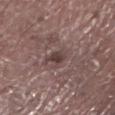Findings:
- workup · total-body-photography surveillance lesion; no biopsy
- automated lesion analysis · a mean CIELAB color near L≈40 a*≈16 b*≈18, roughly 9 lightness units darker than nearby skin, and a normalized border contrast of about 7.5; an automated nevus-likeness rating near 0 out of 100 and lesion-presence confidence of about 95/100
- patient · male, aged 78 to 82
- site · the left lower leg
- image · total-body-photography crop, ~15 mm field of view